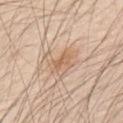– follow-up — catalogued during a skin exam; not biopsied
– body site — the back
– subject — male, approximately 80 years of age
– illumination — white-light
– TBP lesion metrics — border irregularity of about 6.5 on a 0–10 scale and a color-variation rating of about 3.5/10; a classifier nevus-likeness of about 40/100 and lesion-presence confidence of about 95/100
– diameter — ≈4.5 mm
– image — ~15 mm crop, total-body skin-cancer survey